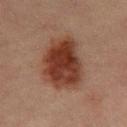Imaged during a routine full-body skin examination; the lesion was not biopsied and no histopathology is available. Measured at roughly 7.5 mm in maximum diameter. The total-body-photography lesion software estimated an area of roughly 25 mm², an eccentricity of roughly 0.8, and a symmetry-axis asymmetry near 0.25. It also reported a mean CIELAB color near L≈30 a*≈19 b*≈23 and a normalized border contrast of about 11.5. The software also gave an automated nevus-likeness rating near 100 out of 100 and a lesion-detection confidence of about 100/100. The patient is a male in their mid- to late 60s. Captured under cross-polarized illumination. A lesion tile, about 15 mm wide, cut from a 3D total-body photograph. Located on the mid back.